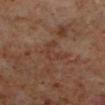This lesion was catalogued during total-body skin photography and was not selected for biopsy. Captured under cross-polarized illumination. A male subject, aged approximately 60. From the left lower leg. A 15 mm crop from a total-body photograph taken for skin-cancer surveillance. Automated tile analysis of the lesion measured internal color variation of about 1 on a 0–10 scale and radial color variation of about 0.5. The software also gave a classifier nevus-likeness of about 0/100.